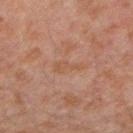This lesion was catalogued during total-body skin photography and was not selected for biopsy. A 15 mm crop from a total-body photograph taken for skin-cancer surveillance. Approximately 3 mm at its widest. The tile uses cross-polarized illumination. From the right forearm. The subject is a male approximately 30 years of age.